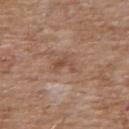The lesion was photographed on a routine skin check and not biopsied; there is no pathology result.
The lesion-visualizer software estimated a lesion area of about 3.5 mm², an eccentricity of roughly 0.75, and a shape-asymmetry score of about 0.5 (0 = symmetric).
A region of skin cropped from a whole-body photographic capture, roughly 15 mm wide.
On the front of the torso.
A male patient, aged 58 to 62.
Measured at roughly 2.5 mm in maximum diameter.
Imaged with white-light lighting.